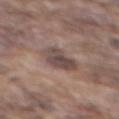Notes:
- workup: total-body-photography surveillance lesion; no biopsy
- lesion diameter: ≈4.5 mm
- body site: the mid back
- subject: male, roughly 75 years of age
- acquisition: total-body-photography crop, ~15 mm field of view
- illumination: white-light